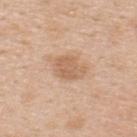<case>
<biopsy_status>not biopsied; imaged during a skin examination</biopsy_status>
<site>back</site>
<patient>
  <sex>male</sex>
  <age_approx>50</age_approx>
</patient>
<lesion_size>
  <long_diameter_mm_approx>3.5</long_diameter_mm_approx>
</lesion_size>
<image>
  <source>total-body photography crop</source>
  <field_of_view_mm>15</field_of_view_mm>
</image>
</case>